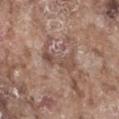Clinical impression: No biopsy was performed on this lesion — it was imaged during a full skin examination and was not determined to be concerning. Image and clinical context: A male subject, in their mid- to late 70s. A 15 mm crop from a total-body photograph taken for skin-cancer surveillance. Automated image analysis of the tile measured a footprint of about 7.5 mm², a shape eccentricity near 0.9, and a symmetry-axis asymmetry near 0.6. It also reported a mean CIELAB color near L≈50 a*≈17 b*≈25, roughly 8 lightness units darker than nearby skin, and a normalized lesion–skin contrast near 6. And it measured a border-irregularity index near 8.5/10, internal color variation of about 4 on a 0–10 scale, and a peripheral color-asymmetry measure near 1.5. The analysis additionally found a classifier nevus-likeness of about 0/100 and lesion-presence confidence of about 70/100. Longest diameter approximately 4.5 mm. The lesion is located on the abdomen.